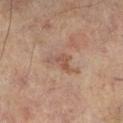Assessment:
The lesion was photographed on a routine skin check and not biopsied; there is no pathology result.
Clinical summary:
The lesion is on the left lower leg. The lesion-visualizer software estimated an average lesion color of about L≈43 a*≈17 b*≈23 (CIELAB), roughly 6 lightness units darker than nearby skin, and a lesion-to-skin contrast of about 5.5 (normalized; higher = more distinct). And it measured a border-irregularity index near 7/10, internal color variation of about 1 on a 0–10 scale, and peripheral color asymmetry of about 0.5. The analysis additionally found a nevus-likeness score of about 0/100 and lesion-presence confidence of about 100/100. A male patient, in their mid- to late 70s. A lesion tile, about 15 mm wide, cut from a 3D total-body photograph. About 3.5 mm across. Imaged with cross-polarized lighting.